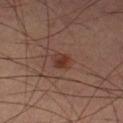{
  "biopsy_status": "not biopsied; imaged during a skin examination",
  "image": {
    "source": "total-body photography crop",
    "field_of_view_mm": 15
  },
  "automated_metrics": {
    "cielab_L": 34,
    "cielab_a": 20,
    "cielab_b": 25,
    "vs_skin_darker_L": 7.0
  },
  "patient": {
    "sex": "male",
    "age_approx": 60
  },
  "site": "left lower leg",
  "lighting": "cross-polarized"
}